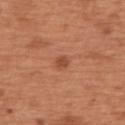Q: Was this lesion biopsied?
A: no biopsy performed (imaged during a skin exam)
Q: What are the patient's age and sex?
A: male, aged 63 to 67
Q: Lesion location?
A: the upper back
Q: How was this image acquired?
A: ~15 mm crop, total-body skin-cancer survey
Q: Illumination type?
A: white-light illumination
Q: How large is the lesion?
A: ~3 mm (longest diameter)
Q: Automated lesion metrics?
A: an eccentricity of roughly 0.8 and two-axis asymmetry of about 0.2; a classifier nevus-likeness of about 75/100 and a lesion-detection confidence of about 100/100